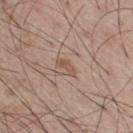Cropped from a total-body skin-imaging series; the visible field is about 15 mm.
About 2.5 mm across.
An algorithmic analysis of the crop reported an area of roughly 2.5 mm², a shape eccentricity near 0.9, and two-axis asymmetry of about 0.4. It also reported an average lesion color of about L≈53 a*≈18 b*≈27 (CIELAB) and about 8 CIELAB-L* units darker than the surrounding skin. And it measured a border-irregularity index near 4/10 and peripheral color asymmetry of about 0.
From the right thigh.
A male subject, aged approximately 60.
The tile uses white-light illumination.